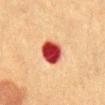A lesion tile, about 15 mm wide, cut from a 3D total-body photograph. About 3.5 mm across. Automated image analysis of the tile measured a mean CIELAB color near L≈40 a*≈35 b*≈29, a lesion–skin lightness drop of about 22, and a normalized border contrast of about 16. It also reported a border-irregularity rating of about 1/10 and a within-lesion color-variation index near 5/10. The lesion is located on the abdomen. The patient is a male aged approximately 50.